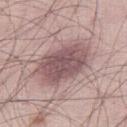Assessment:
Part of a total-body skin-imaging series; this lesion was reviewed on a skin check and was not flagged for biopsy.
Background:
An algorithmic analysis of the crop reported a lesion area of about 22 mm², a shape eccentricity near 0.8, and a shape-asymmetry score of about 0.15 (0 = symmetric). The software also gave a mean CIELAB color near L≈53 a*≈19 b*≈17, about 13 CIELAB-L* units darker than the surrounding skin, and a normalized border contrast of about 9. And it measured an automated nevus-likeness rating near 40 out of 100 and a lesion-detection confidence of about 100/100. The recorded lesion diameter is about 7 mm. Cropped from a total-body skin-imaging series; the visible field is about 15 mm. A male patient, roughly 50 years of age. Located on the right thigh.